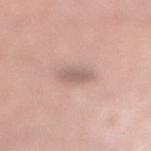{
  "biopsy_status": "not biopsied; imaged during a skin examination",
  "lesion_size": {
    "long_diameter_mm_approx": 2.5
  },
  "image": {
    "source": "total-body photography crop",
    "field_of_view_mm": 15
  },
  "patient": {
    "sex": "female",
    "age_approx": 30
  },
  "lighting": "white-light",
  "automated_metrics": {
    "border_irregularity_0_10": 1.5,
    "color_variation_0_10": 1.5
  },
  "site": "right lower leg"
}